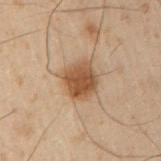Case summary:
* workup · total-body-photography surveillance lesion; no biopsy
* TBP lesion metrics · an average lesion color of about L≈42 a*≈16 b*≈29 (CIELAB), about 11 CIELAB-L* units darker than the surrounding skin, and a lesion-to-skin contrast of about 9.5 (normalized; higher = more distinct); a nevus-likeness score of about 100/100 and a detector confidence of about 100 out of 100 that the crop contains a lesion
* subject · male, aged around 50
* lesion diameter · about 4 mm
* body site · the left arm
* acquisition · ~15 mm tile from a whole-body skin photo